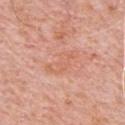  biopsy_status: not biopsied; imaged during a skin examination
  site: front of the torso
  lesion_size:
    long_diameter_mm_approx: 4.5
  image:
    source: total-body photography crop
    field_of_view_mm: 15
  patient:
    sex: male
    age_approx: 80